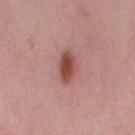Clinical impression: The lesion was tiled from a total-body skin photograph and was not biopsied. Acquisition and patient details: From the right thigh. A 15 mm close-up extracted from a 3D total-body photography capture. A female patient aged around 50. This is a white-light tile. Longest diameter approximately 3.5 mm.